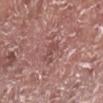Assessment:
The lesion was tiled from a total-body skin photograph and was not biopsied.
Background:
A roughly 15 mm field-of-view crop from a total-body skin photograph. Automated image analysis of the tile measured an area of roughly 4 mm², a shape eccentricity near 0.75, and a shape-asymmetry score of about 0.35 (0 = symmetric). And it measured a within-lesion color-variation index near 3/10 and peripheral color asymmetry of about 1. On the right lower leg. Measured at roughly 2.5 mm in maximum diameter. The patient is a male aged approximately 75.